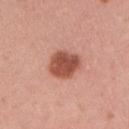The lesion was photographed on a routine skin check and not biopsied; there is no pathology result. On the right upper arm. Captured under white-light illumination. The lesion-visualizer software estimated a lesion area of about 11 mm² and a shape eccentricity near 0.55. And it measured a mean CIELAB color near L≈52 a*≈28 b*≈30, about 15 CIELAB-L* units darker than the surrounding skin, and a lesion-to-skin contrast of about 10 (normalized; higher = more distinct). And it measured border irregularity of about 1 on a 0–10 scale and peripheral color asymmetry of about 1. The software also gave a detector confidence of about 100 out of 100 that the crop contains a lesion. A female patient, in their mid- to late 20s. A region of skin cropped from a whole-body photographic capture, roughly 15 mm wide.